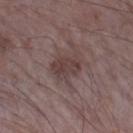biopsy_status: not biopsied; imaged during a skin examination
image:
  source: total-body photography crop
  field_of_view_mm: 15
automated_metrics:
  vs_skin_darker_L: 8.0
  vs_skin_contrast_norm: 7.0
lesion_size:
  long_diameter_mm_approx: 3.5
lighting: white-light
site: left forearm
patient:
  sex: male
  age_approx: 65